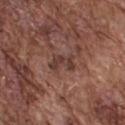Clinical impression: Captured during whole-body skin photography for melanoma surveillance; the lesion was not biopsied. Image and clinical context: The recorded lesion diameter is about 3.5 mm. A male patient, aged 73–77. Imaged with white-light lighting. A 15 mm crop from a total-body photograph taken for skin-cancer surveillance. The lesion is located on the upper back. Automated image analysis of the tile measured an average lesion color of about L≈38 a*≈20 b*≈23 (CIELAB), about 8 CIELAB-L* units darker than the surrounding skin, and a normalized lesion–skin contrast near 7.